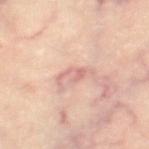  biopsy_status: not biopsied; imaged during a skin examination
  lesion_size:
    long_diameter_mm_approx: 3.5
  image:
    source: total-body photography crop
    field_of_view_mm: 15
  site: right thigh
  automated_metrics:
    cielab_L: 66
    cielab_a: 23
    cielab_b: 25
    vs_skin_darker_L: 9.0
    vs_skin_contrast_norm: 5.5
    border_irregularity_0_10: 8.0
    color_variation_0_10: 0.5
    peripheral_color_asymmetry: 0.0
    nevus_likeness_0_100: 0
    lesion_detection_confidence_0_100: 95
  patient:
    sex: female
    age_approx: 45
  lighting: cross-polarized